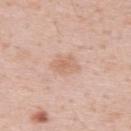Image and clinical context: The lesion is on the upper back. The subject is a male aged approximately 35. A 15 mm crop from a total-body photograph taken for skin-cancer surveillance. The recorded lesion diameter is about 3 mm. Captured under white-light illumination.